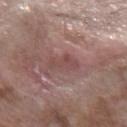{"biopsy_status": "not biopsied; imaged during a skin examination", "automated_metrics": {"vs_skin_darker_L": 7.0, "vs_skin_contrast_norm": 5.5, "border_irregularity_0_10": 5.0, "color_variation_0_10": 1.5, "peripheral_color_asymmetry": 0.5}, "patient": {"sex": "male", "age_approx": 60}, "site": "left forearm", "lesion_size": {"long_diameter_mm_approx": 3.5}, "lighting": "white-light", "image": {"source": "total-body photography crop", "field_of_view_mm": 15}}